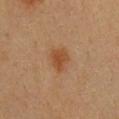{"biopsy_status": "not biopsied; imaged during a skin examination", "site": "chest", "lighting": "cross-polarized", "patient": {"sex": "female", "age_approx": 40}, "image": {"source": "total-body photography crop", "field_of_view_mm": 15}}